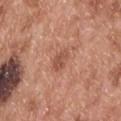Recorded during total-body skin imaging; not selected for excision or biopsy. The lesion-visualizer software estimated an area of roughly 4.5 mm² and two-axis asymmetry of about 0.2. The software also gave a lesion color around L≈53 a*≈24 b*≈31 in CIELAB and a lesion-to-skin contrast of about 6 (normalized; higher = more distinct). It also reported border irregularity of about 1.5 on a 0–10 scale, a color-variation rating of about 2.5/10, and a peripheral color-asymmetry measure near 1. The analysis additionally found an automated nevus-likeness rating near 15 out of 100 and a detector confidence of about 100 out of 100 that the crop contains a lesion. A male patient about 55 years old. A close-up tile cropped from a whole-body skin photograph, about 15 mm across. From the upper back.